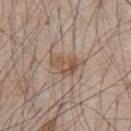Clinical impression:
Captured during whole-body skin photography for melanoma surveillance; the lesion was not biopsied.
Background:
A roughly 15 mm field-of-view crop from a total-body skin photograph. A male subject aged 43 to 47. From the chest. Imaged with white-light lighting.